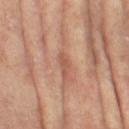patient: female, aged approximately 75
image source: ~15 mm tile from a whole-body skin photo
body site: the left thigh